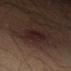<lesion>
<biopsy_status>not biopsied; imaged during a skin examination</biopsy_status>
<lesion_size>
  <long_diameter_mm_approx>4.0</long_diameter_mm_approx>
</lesion_size>
<automated_metrics>
  <border_irregularity_0_10>2.5</border_irregularity_0_10>
  <nevus_likeness_0_100>15</nevus_likeness_0_100>
  <lesion_detection_confidence_0_100>100</lesion_detection_confidence_0_100>
</automated_metrics>
<site>left thigh</site>
<patient>
  <sex>male</sex>
  <age_approx>35</age_approx>
</patient>
<image>
  <source>total-body photography crop</source>
  <field_of_view_mm>15</field_of_view_mm>
</image>
<lighting>cross-polarized</lighting>
</lesion>